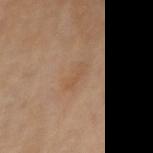Q: Was this lesion biopsied?
A: imaged on a skin check; not biopsied
Q: What are the patient's age and sex?
A: female, aged 68 to 72
Q: What kind of image is this?
A: ~15 mm tile from a whole-body skin photo
Q: Lesion size?
A: about 3 mm
Q: What is the anatomic site?
A: the left forearm
Q: What did automated image analysis measure?
A: an average lesion color of about L≈53 a*≈18 b*≈34 (CIELAB), about 6 CIELAB-L* units darker than the surrounding skin, and a lesion-to-skin contrast of about 5 (normalized; higher = more distinct); a color-variation rating of about 0/10 and peripheral color asymmetry of about 0; a nevus-likeness score of about 0/100